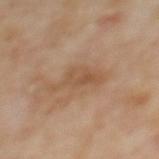Impression: This lesion was catalogued during total-body skin photography and was not selected for biopsy. Context: A female subject aged 58–62. Imaged with cross-polarized lighting. The lesion is located on the upper back. A close-up tile cropped from a whole-body skin photograph, about 15 mm across.